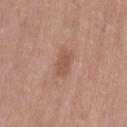Notes:
* workup · total-body-photography surveillance lesion; no biopsy
* diameter · about 3 mm
* subject · female, aged around 40
* lighting · white-light illumination
* location · the left thigh
* image · 15 mm crop, total-body photography
* image-analysis metrics · border irregularity of about 2 on a 0–10 scale and a color-variation rating of about 1.5/10; a nevus-likeness score of about 0/100 and lesion-presence confidence of about 100/100